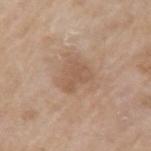* workup: imaged on a skin check; not biopsied
* subject: female, aged around 75
* acquisition: 15 mm crop, total-body photography
* site: the arm
* diameter: ≈3.5 mm
* lighting: white-light